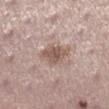<tbp_lesion>
<lesion_size>
  <long_diameter_mm_approx>3.5</long_diameter_mm_approx>
</lesion_size>
<image>
  <source>total-body photography crop</source>
  <field_of_view_mm>15</field_of_view_mm>
</image>
<site>right lower leg</site>
<lighting>white-light</lighting>
<automated_metrics>
  <cielab_L>55</cielab_L>
  <cielab_a>17</cielab_a>
  <cielab_b>23</cielab_b>
  <vs_skin_darker_L>10.0</vs_skin_darker_L>
  <vs_skin_contrast_norm>7.5</vs_skin_contrast_norm>
</automated_metrics>
<patient>
  <sex>female</sex>
  <age_approx>25</age_approx>
</patient>
</tbp_lesion>